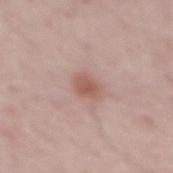automated lesion analysis: a shape eccentricity near 0.6; an average lesion color of about L≈56 a*≈21 b*≈26 (CIELAB) and roughly 9 lightness units darker than nearby skin; a border-irregularity rating of about 2/10, a within-lesion color-variation index near 2/10, and peripheral color asymmetry of about 0.5; an automated nevus-likeness rating near 90 out of 100 and a lesion-detection confidence of about 100/100
location: the mid back
image source: ~15 mm tile from a whole-body skin photo
patient: male, about 50 years old